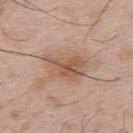Imaged during a routine full-body skin examination; the lesion was not biopsied and no histopathology is available. The subject is a male in their mid- to late 50s. Cropped from a whole-body photographic skin survey; the tile spans about 15 mm. The lesion is located on the upper back.